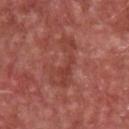The lesion was tiled from a total-body skin photograph and was not biopsied. The lesion is on the front of the torso. This is a white-light tile. A male subject about 65 years old. Cropped from a total-body skin-imaging series; the visible field is about 15 mm.